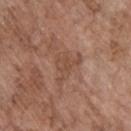Findings:
- notes: total-body-photography surveillance lesion; no biopsy
- image source: ~15 mm crop, total-body skin-cancer survey
- TBP lesion metrics: roughly 7 lightness units darker than nearby skin and a lesion-to-skin contrast of about 5.5 (normalized; higher = more distinct); a classifier nevus-likeness of about 0/100
- illumination: white-light illumination
- subject: male, approximately 70 years of age
- body site: the upper back